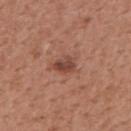biopsy status: no biopsy performed (imaged during a skin exam) | TBP lesion metrics: a footprint of about 5 mm² and an eccentricity of roughly 0.65; a border-irregularity rating of about 2/10 and a peripheral color-asymmetry measure near 1; a lesion-detection confidence of about 100/100 | anatomic site: the mid back | imaging modality: ~15 mm tile from a whole-body skin photo | tile lighting: white-light illumination | subject: male, about 55 years old.